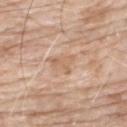{"biopsy_status": "not biopsied; imaged during a skin examination", "site": "upper back", "image": {"source": "total-body photography crop", "field_of_view_mm": 15}, "automated_metrics": {"border_irregularity_0_10": 5.0, "color_variation_0_10": 1.5, "peripheral_color_asymmetry": 0.5}, "lighting": "white-light", "patient": {"sex": "male", "age_approx": 75}, "lesion_size": {"long_diameter_mm_approx": 3.0}}